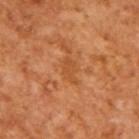{
  "patient": {
    "sex": "male",
    "age_approx": 65
  },
  "image": {
    "source": "total-body photography crop",
    "field_of_view_mm": 15
  }
}